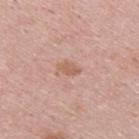{"biopsy_status": "not biopsied; imaged during a skin examination", "lighting": "white-light", "automated_metrics": {"area_mm2_approx": 3.0, "eccentricity": 0.85, "shape_asymmetry": 0.2, "border_irregularity_0_10": 2.5, "color_variation_0_10": 0.5, "peripheral_color_asymmetry": 0.5}, "patient": {"sex": "male", "age_approx": 25}, "lesion_size": {"long_diameter_mm_approx": 2.5}, "site": "upper back", "image": {"source": "total-body photography crop", "field_of_view_mm": 15}}